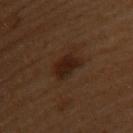follow-up=total-body-photography surveillance lesion; no biopsy
lesion size=≈4 mm
location=the upper back
tile lighting=cross-polarized
acquisition=~15 mm tile from a whole-body skin photo
subject=female, aged around 50